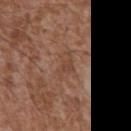{"biopsy_status": "not biopsied; imaged during a skin examination", "image": {"source": "total-body photography crop", "field_of_view_mm": 15}, "site": "chest", "patient": {"sex": "male", "age_approx": 45}, "lighting": "white-light"}